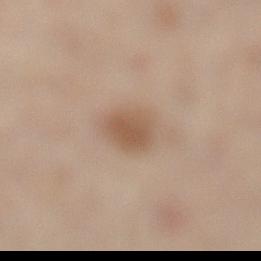Notes:
– lighting: cross-polarized
– acquisition: total-body-photography crop, ~15 mm field of view
– anatomic site: the left lower leg
– TBP lesion metrics: an area of roughly 6 mm², an eccentricity of roughly 0.65, and a symmetry-axis asymmetry near 0.25; a border-irregularity index near 2/10, a color-variation rating of about 2/10, and radial color variation of about 0.5; an automated nevus-likeness rating near 80 out of 100
– size: ~3 mm (longest diameter)
– patient: female, aged approximately 55